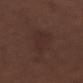No biopsy was performed on this lesion — it was imaged during a full skin examination and was not determined to be concerning.
Cropped from a total-body skin-imaging series; the visible field is about 15 mm.
Imaged with white-light lighting.
A male subject aged around 50.
The lesion is on the right forearm.